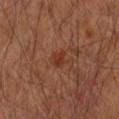Q: How was this image acquired?
A: total-body-photography crop, ~15 mm field of view
Q: What are the patient's age and sex?
A: male, approximately 65 years of age
Q: Lesion location?
A: the right upper arm
Q: How was the tile lit?
A: cross-polarized illumination
Q: What did automated image analysis measure?
A: a lesion area of about 2 mm²; an average lesion color of about L≈33 a*≈25 b*≈31 (CIELAB) and a normalized lesion–skin contrast near 7.5; border irregularity of about 2 on a 0–10 scale and internal color variation of about 0.5 on a 0–10 scale